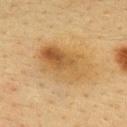• biopsy status: total-body-photography surveillance lesion; no biopsy
• anatomic site: the back
• subject: female, approximately 40 years of age
• imaging modality: ~15 mm crop, total-body skin-cancer survey